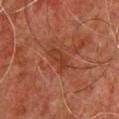A male subject aged 58–62. From the chest. Approximately 3.5 mm at its widest. Captured under cross-polarized illumination. A lesion tile, about 15 mm wide, cut from a 3D total-body photograph. An algorithmic analysis of the crop reported an eccentricity of roughly 0.85 and two-axis asymmetry of about 0.35. The analysis additionally found a lesion–skin lightness drop of about 5 and a normalized lesion–skin contrast near 6. The analysis additionally found a nevus-likeness score of about 0/100 and a detector confidence of about 100 out of 100 that the crop contains a lesion.